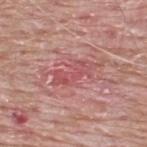Findings:
- notes: total-body-photography surveillance lesion; no biopsy
- TBP lesion metrics: an area of roughly 8.5 mm² and a shape-asymmetry score of about 0.45 (0 = symmetric); a border-irregularity rating of about 6/10, internal color variation of about 4 on a 0–10 scale, and peripheral color asymmetry of about 1.5; an automated nevus-likeness rating near 0 out of 100 and a lesion-detection confidence of about 70/100
- body site: the upper back
- subject: male, in their mid- to late 60s
- image source: ~15 mm tile from a whole-body skin photo
- diameter: ≈5 mm
- lighting: white-light